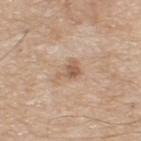acquisition: ~15 mm crop, total-body skin-cancer survey | automated lesion analysis: a footprint of about 4 mm², a shape eccentricity near 0.75, and a symmetry-axis asymmetry near 0.45; roughly 10 lightness units darker than nearby skin and a normalized lesion–skin contrast near 7; a border-irregularity rating of about 5/10, a color-variation rating of about 3/10, and radial color variation of about 1 | tile lighting: white-light | lesion size: about 3 mm | subject: male, aged around 70 | body site: the back.